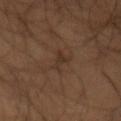{
  "biopsy_status": "not biopsied; imaged during a skin examination",
  "lesion_size": {
    "long_diameter_mm_approx": 3.5
  },
  "automated_metrics": {
    "area_mm2_approx": 3.5,
    "eccentricity": 0.9,
    "border_irregularity_0_10": 7.5
  },
  "image": {
    "source": "total-body photography crop",
    "field_of_view_mm": 15
  },
  "lighting": "cross-polarized",
  "patient": {
    "sex": "male",
    "age_approx": 60
  },
  "site": "abdomen"
}